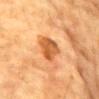Imaged during a routine full-body skin examination; the lesion was not biopsied and no histopathology is available. On the chest. Measured at roughly 3.5 mm in maximum diameter. The tile uses cross-polarized illumination. A roughly 15 mm field-of-view crop from a total-body skin photograph. A male subject, about 85 years old or older.